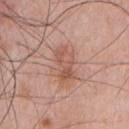Q: Was a biopsy performed?
A: imaged on a skin check; not biopsied
Q: Lesion size?
A: ≈4.5 mm
Q: What is the anatomic site?
A: the chest
Q: What are the patient's age and sex?
A: male, roughly 50 years of age
Q: How was this image acquired?
A: 15 mm crop, total-body photography